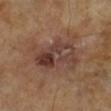workup — total-body-photography surveillance lesion; no biopsy
subject — male, in their mid- to late 60s
image — 15 mm crop, total-body photography